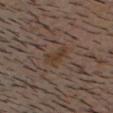Clinical impression: The lesion was tiled from a total-body skin photograph and was not biopsied. Acquisition and patient details: A lesion tile, about 15 mm wide, cut from a 3D total-body photograph. Located on the head or neck. A male subject, aged approximately 40.